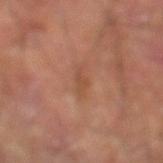{"biopsy_status": "not biopsied; imaged during a skin examination", "patient": {"sex": "male", "age_approx": 70}, "lighting": "cross-polarized", "site": "left lower leg", "image": {"source": "total-body photography crop", "field_of_view_mm": 15}}